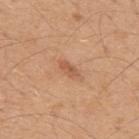Assessment:
The lesion was tiled from a total-body skin photograph and was not biopsied.
Acquisition and patient details:
A close-up tile cropped from a whole-body skin photograph, about 15 mm across. The lesion is located on the mid back. The subject is a male approximately 50 years of age.